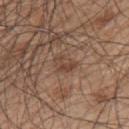Recorded during total-body skin imaging; not selected for excision or biopsy. A male patient, aged 48–52. On the back. Approximately 3 mm at its widest. The tile uses white-light illumination. A region of skin cropped from a whole-body photographic capture, roughly 15 mm wide. The lesion-visualizer software estimated an eccentricity of roughly 0.6 and two-axis asymmetry of about 0.35. And it measured a lesion-to-skin contrast of about 5.5 (normalized; higher = more distinct). The software also gave a border-irregularity rating of about 3/10, a color-variation rating of about 3.5/10, and peripheral color asymmetry of about 1.5. The software also gave a classifier nevus-likeness of about 0/100 and a detector confidence of about 100 out of 100 that the crop contains a lesion.